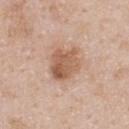Q: Lesion size?
A: about 3.5 mm
Q: Who is the patient?
A: male, approximately 40 years of age
Q: How was this image acquired?
A: ~15 mm tile from a whole-body skin photo
Q: What lighting was used for the tile?
A: white-light
Q: Lesion location?
A: the back